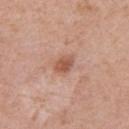Impression: Captured during whole-body skin photography for melanoma surveillance; the lesion was not biopsied. Context: A female patient roughly 60 years of age. A region of skin cropped from a whole-body photographic capture, roughly 15 mm wide. Measured at roughly 2.5 mm in maximum diameter. On the right upper arm.